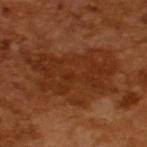The lesion was photographed on a routine skin check and not biopsied; there is no pathology result.
A 15 mm crop from a total-body photograph taken for skin-cancer surveillance.
An algorithmic analysis of the crop reported an area of roughly 39 mm², an outline eccentricity of about 0.85 (0 = round, 1 = elongated), and two-axis asymmetry of about 0.35. It also reported a color-variation rating of about 3/10 and a peripheral color-asymmetry measure near 1. The analysis additionally found a lesion-detection confidence of about 90/100.
A male subject aged 63 to 67.
Measured at roughly 10 mm in maximum diameter.
Captured under cross-polarized illumination.